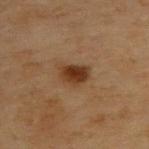Imaged during a routine full-body skin examination; the lesion was not biopsied and no histopathology is available.
Imaged with cross-polarized lighting.
A 15 mm close-up tile from a total-body photography series done for melanoma screening.
On the upper back.
A male patient roughly 55 years of age.